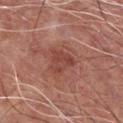This lesion was catalogued during total-body skin photography and was not selected for biopsy.
Automated tile analysis of the lesion measured a lesion area of about 6 mm².
About 3 mm across.
A 15 mm close-up tile from a total-body photography series done for melanoma screening.
A male subject about 65 years old.
On the chest.
Captured under white-light illumination.